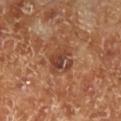{"biopsy_status": "not biopsied; imaged during a skin examination", "image": {"source": "total-body photography crop", "field_of_view_mm": 15}, "site": "left lower leg", "patient": {"sex": "male", "age_approx": 70}}